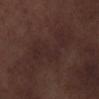| feature | finding |
|---|---|
| workup | imaged on a skin check; not biopsied |
| automated lesion analysis | roughly 3 lightness units darker than nearby skin and a normalized border contrast of about 4.5; a border-irregularity rating of about 9/10, a within-lesion color-variation index near 2/10, and radial color variation of about 1 |
| acquisition | 15 mm crop, total-body photography |
| location | the left lower leg |
| lighting | white-light |
| patient | male, roughly 70 years of age |
| diameter | about 8 mm |